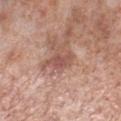<record>
<lesion_size>
  <long_diameter_mm_approx>3.0</long_diameter_mm_approx>
</lesion_size>
<image>
  <source>total-body photography crop</source>
  <field_of_view_mm>15</field_of_view_mm>
</image>
<patient>
  <sex>male</sex>
  <age_approx>55</age_approx>
</patient>
<lighting>white-light</lighting>
<site>leg</site>
</record>